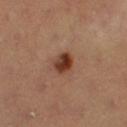notes — total-body-photography surveillance lesion; no biopsy | lesion diameter — ~3 mm (longest diameter) | body site — the right lower leg | image — total-body-photography crop, ~15 mm field of view | image-analysis metrics — border irregularity of about 1.5 on a 0–10 scale, internal color variation of about 6.5 on a 0–10 scale, and peripheral color asymmetry of about 2; lesion-presence confidence of about 100/100 | tile lighting — cross-polarized illumination | patient — female, approximately 35 years of age.